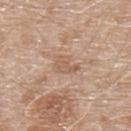  image:
    source: total-body photography crop
    field_of_view_mm: 15
  patient:
    sex: male
    age_approx: 80
  site: upper back